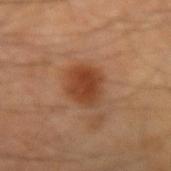Impression:
The lesion was tiled from a total-body skin photograph and was not biopsied.
Context:
The lesion's longest dimension is about 4 mm. Imaged with cross-polarized lighting. The patient is a male aged 43–47. A 15 mm close-up tile from a total-body photography series done for melanoma screening. The lesion is on the left forearm. Automated tile analysis of the lesion measured a footprint of about 11 mm² and two-axis asymmetry of about 0.15. It also reported a mean CIELAB color near L≈40 a*≈23 b*≈32, about 10 CIELAB-L* units darker than the surrounding skin, and a lesion-to-skin contrast of about 8.5 (normalized; higher = more distinct).